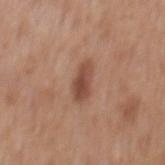A region of skin cropped from a whole-body photographic capture, roughly 15 mm wide. The recorded lesion diameter is about 4 mm. A male patient in their 60s. Captured under white-light illumination. On the mid back.